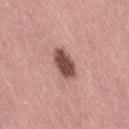Clinical impression:
This lesion was catalogued during total-body skin photography and was not selected for biopsy.
Background:
The recorded lesion diameter is about 4 mm. Imaged with white-light lighting. Located on the lower back. A close-up tile cropped from a whole-body skin photograph, about 15 mm across. A female subject, aged approximately 55.